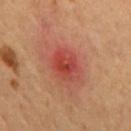{"biopsy_status": "not biopsied; imaged during a skin examination", "automated_metrics": {"cielab_L": 43, "cielab_a": 31, "cielab_b": 28, "vs_skin_contrast_norm": 7.0, "nevus_likeness_0_100": 0, "lesion_detection_confidence_0_100": 100}, "lesion_size": {"long_diameter_mm_approx": 4.5}, "site": "mid back", "patient": {"sex": "male", "age_approx": 55}, "image": {"source": "total-body photography crop", "field_of_view_mm": 15}, "lighting": "cross-polarized"}